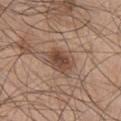A male patient, roughly 45 years of age. The tile uses white-light illumination. On the chest. The lesion's longest dimension is about 4.5 mm. A lesion tile, about 15 mm wide, cut from a 3D total-body photograph.